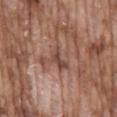site = the mid back | subject = male, approximately 75 years of age | imaging modality = ~15 mm tile from a whole-body skin photo.